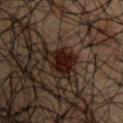The lesion was photographed on a routine skin check and not biopsied; there is no pathology result. A male subject in their 50s. The lesion is located on the arm. A close-up tile cropped from a whole-body skin photograph, about 15 mm across.